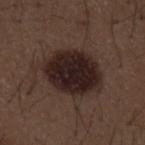Recorded during total-body skin imaging; not selected for excision or biopsy. Located on the back. A male subject roughly 50 years of age. A 15 mm crop from a total-body photograph taken for skin-cancer surveillance.